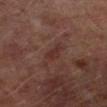From the left lower leg.
This image is a 15 mm lesion crop taken from a total-body photograph.
Approximately 2.5 mm at its widest.
A male patient in their mid- to late 60s.